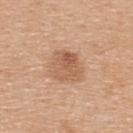anatomic site: the upper back
automated lesion analysis: a footprint of about 9.5 mm², an eccentricity of roughly 0.55, and a symmetry-axis asymmetry near 0.15; a mean CIELAB color near L≈58 a*≈21 b*≈33, a lesion–skin lightness drop of about 9, and a lesion-to-skin contrast of about 6.5 (normalized; higher = more distinct); an automated nevus-likeness rating near 35 out of 100
patient: female, approximately 45 years of age
diameter: ≈4 mm
illumination: white-light illumination
acquisition: ~15 mm crop, total-body skin-cancer survey